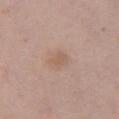Clinical impression:
Recorded during total-body skin imaging; not selected for excision or biopsy.
Image and clinical context:
Imaged with white-light lighting. The lesion is located on the chest. Longest diameter approximately 2.5 mm. The patient is a female in their 40s. The total-body-photography lesion software estimated an average lesion color of about L≈58 a*≈17 b*≈28 (CIELAB). A close-up tile cropped from a whole-body skin photograph, about 15 mm across.